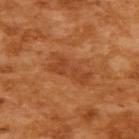Clinical impression: The lesion was tiled from a total-body skin photograph and was not biopsied. Background: From the upper back. Longest diameter approximately 5 mm. Imaged with cross-polarized lighting. Automated image analysis of the tile measured a lesion area of about 8.5 mm², an eccentricity of roughly 0.95, and a symmetry-axis asymmetry near 0.35. The analysis additionally found an average lesion color of about L≈46 a*≈29 b*≈40 (CIELAB), roughly 7 lightness units darker than nearby skin, and a normalized lesion–skin contrast near 5.5. The software also gave a nevus-likeness score of about 15/100 and a lesion-detection confidence of about 100/100. The patient is a female aged 53 to 57. A 15 mm crop from a total-body photograph taken for skin-cancer surveillance.